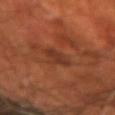The lesion was photographed on a routine skin check and not biopsied; there is no pathology result. Automated image analysis of the tile measured a footprint of about 4 mm² and a symmetry-axis asymmetry near 0.35. Captured under cross-polarized illumination. A 15 mm close-up extracted from a 3D total-body photography capture. The lesion is located on the arm. A male subject aged around 70.